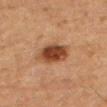<lesion>
<biopsy_status>not biopsied; imaged during a skin examination</biopsy_status>
<site>leg</site>
<lighting>cross-polarized</lighting>
<lesion_size>
  <long_diameter_mm_approx>4.5</long_diameter_mm_approx>
</lesion_size>
<automated_metrics>
  <area_mm2_approx>10.0</area_mm2_approx>
  <eccentricity>0.75</eccentricity>
  <border_irregularity_0_10>1.5</border_irregularity_0_10>
  <color_variation_0_10>4.0</color_variation_0_10>
  <peripheral_color_asymmetry>1.0</peripheral_color_asymmetry>
</automated_metrics>
<image>
  <source>total-body photography crop</source>
  <field_of_view_mm>15</field_of_view_mm>
</image>
<patient>
  <sex>male</sex>
  <age_approx>75</age_approx>
</patient>
</lesion>